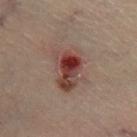From the right lower leg. An algorithmic analysis of the crop reported a border-irregularity rating of about 3.5/10 and radial color variation of about 3.5. A lesion tile, about 15 mm wide, cut from a 3D total-body photograph. A male patient aged 53–57.